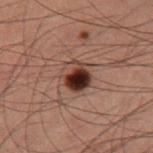Captured during whole-body skin photography for melanoma surveillance; the lesion was not biopsied. The tile uses cross-polarized illumination. The total-body-photography lesion software estimated internal color variation of about 10 on a 0–10 scale and a peripheral color-asymmetry measure near 5. On the right thigh. The patient is a male about 60 years old. Measured at roughly 3.5 mm in maximum diameter. A roughly 15 mm field-of-view crop from a total-body skin photograph.